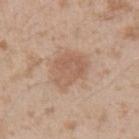Notes:
– notes · no biopsy performed (imaged during a skin exam)
– lesion diameter · ≈5 mm
– subject · male, aged 23 to 27
– anatomic site · the left upper arm
– acquisition · ~15 mm tile from a whole-body skin photo
– illumination · white-light